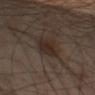{"biopsy_status": "not biopsied; imaged during a skin examination", "patient": {"sex": "male", "age_approx": 55}, "automated_metrics": {"eccentricity": 0.85, "shape_asymmetry": 0.25, "cielab_L": 26, "cielab_a": 12, "cielab_b": 20, "vs_skin_contrast_norm": 8.5, "border_irregularity_0_10": 3.0, "peripheral_color_asymmetry": 1.0, "lesion_detection_confidence_0_100": 100}, "image": {"source": "total-body photography crop", "field_of_view_mm": 15}, "lighting": "cross-polarized", "lesion_size": {"long_diameter_mm_approx": 4.5}, "site": "left thigh"}